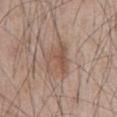No biopsy was performed on this lesion — it was imaged during a full skin examination and was not determined to be concerning. Automated tile analysis of the lesion measured internal color variation of about 3 on a 0–10 scale. From the front of the torso. A 15 mm close-up tile from a total-body photography series done for melanoma screening. The tile uses white-light illumination. A male patient in their mid-60s. Approximately 4 mm at its widest.